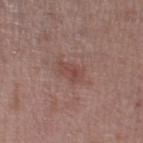Part of a total-body skin-imaging series; this lesion was reviewed on a skin check and was not flagged for biopsy.